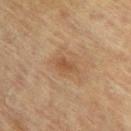No biopsy was performed on this lesion — it was imaged during a full skin examination and was not determined to be concerning. A 15 mm crop from a total-body photograph taken for skin-cancer surveillance. A female subject approximately 80 years of age. Located on the upper back.